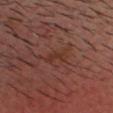Clinical impression: The lesion was photographed on a routine skin check and not biopsied; there is no pathology result. Acquisition and patient details: Located on the head or neck. This is a cross-polarized tile. A roughly 15 mm field-of-view crop from a total-body skin photograph. A male patient, approximately 40 years of age. The lesion-visualizer software estimated a footprint of about 4 mm², a shape eccentricity near 0.8, and two-axis asymmetry of about 0.35. And it measured roughly 5 lightness units darker than nearby skin.